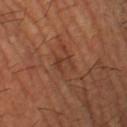workup = imaged on a skin check; not biopsied | image source = ~15 mm tile from a whole-body skin photo | body site = the left lower leg | automated lesion analysis = border irregularity of about 7 on a 0–10 scale and peripheral color asymmetry of about 0 | lighting = cross-polarized illumination | patient = male, aged around 65 | lesion diameter = about 2.5 mm.